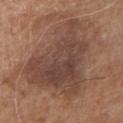| key | value |
|---|---|
| TBP lesion metrics | a border-irregularity index near 6/10, internal color variation of about 4.5 on a 0–10 scale, and a peripheral color-asymmetry measure near 1.5; a nevus-likeness score of about 0/100 and a lesion-detection confidence of about 95/100 |
| lesion diameter | ≈10 mm |
| patient | male, aged 68–72 |
| illumination | white-light |
| location | the chest |
| image | ~15 mm tile from a whole-body skin photo |